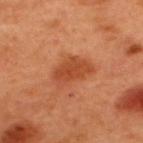This lesion was catalogued during total-body skin photography and was not selected for biopsy.
A region of skin cropped from a whole-body photographic capture, roughly 15 mm wide.
This is a cross-polarized tile.
The patient is a male aged 48 to 52.
On the upper back.